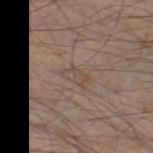Q: Was this lesion biopsied?
A: total-body-photography surveillance lesion; no biopsy
Q: What kind of image is this?
A: ~15 mm tile from a whole-body skin photo
Q: How large is the lesion?
A: ≈3 mm
Q: Patient demographics?
A: male, in their mid- to late 50s
Q: Automated lesion metrics?
A: a symmetry-axis asymmetry near 0.3
Q: Where on the body is the lesion?
A: the leg